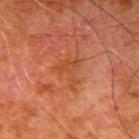lighting: cross-polarized
automated_metrics:
  area_mm2_approx: 6.5
  shape_asymmetry: 0.7
  nevus_likeness_0_100: 0
  lesion_detection_confidence_0_100: 100
patient:
  sex: male
  age_approx: 80
image:
  source: total-body photography crop
  field_of_view_mm: 15
site: right upper arm
lesion_size:
  long_diameter_mm_approx: 4.0